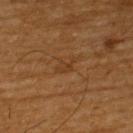notes: imaged on a skin check; not biopsied | illumination: cross-polarized illumination | subject: male, aged 58–62 | automated lesion analysis: a footprint of about 3 mm², an outline eccentricity of about 0.85 (0 = round, 1 = elongated), and a shape-asymmetry score of about 0.45 (0 = symmetric); an average lesion color of about L≈34 a*≈19 b*≈33 (CIELAB), a lesion–skin lightness drop of about 5, and a normalized lesion–skin contrast near 5 | body site: the back | image source: total-body-photography crop, ~15 mm field of view | diameter: ~3 mm (longest diameter).